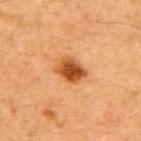Q: Is there a histopathology result?
A: imaged on a skin check; not biopsied
Q: Where on the body is the lesion?
A: the upper back
Q: How large is the lesion?
A: ~3.5 mm (longest diameter)
Q: What is the imaging modality?
A: 15 mm crop, total-body photography
Q: What did automated image analysis measure?
A: an area of roughly 7.5 mm², an outline eccentricity of about 0.7 (0 = round, 1 = elongated), and a symmetry-axis asymmetry near 0.2; a nevus-likeness score of about 100/100 and a lesion-detection confidence of about 100/100
Q: What are the patient's age and sex?
A: male, about 65 years old